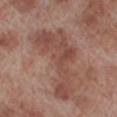This lesion was catalogued during total-body skin photography and was not selected for biopsy.
The lesion is located on the left lower leg.
An algorithmic analysis of the crop reported an average lesion color of about L≈47 a*≈21 b*≈25 (CIELAB) and a lesion-to-skin contrast of about 6.5 (normalized; higher = more distinct). It also reported a nevus-likeness score of about 0/100 and a detector confidence of about 95 out of 100 that the crop contains a lesion.
The lesion's longest dimension is about 10 mm.
This is a white-light tile.
A male subject aged 68–72.
A 15 mm close-up extracted from a 3D total-body photography capture.